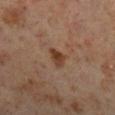{
  "biopsy_status": "not biopsied; imaged during a skin examination",
  "lighting": "cross-polarized",
  "lesion_size": {
    "long_diameter_mm_approx": 2.5
  },
  "image": {
    "source": "total-body photography crop",
    "field_of_view_mm": 15
  },
  "site": "right lower leg",
  "automated_metrics": {
    "cielab_L": 36,
    "cielab_a": 18,
    "cielab_b": 28,
    "vs_skin_darker_L": 9.0,
    "border_irregularity_0_10": 3.5,
    "color_variation_0_10": 3.5,
    "peripheral_color_asymmetry": 1.0
  },
  "patient": {
    "sex": "male",
    "age_approx": 60
  }
}